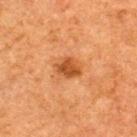Part of a total-body skin-imaging series; this lesion was reviewed on a skin check and was not flagged for biopsy. A close-up tile cropped from a whole-body skin photograph, about 15 mm across. Approximately 3 mm at its widest. Located on the back. The subject is a male in their 50s. Automated tile analysis of the lesion measured an average lesion color of about L≈41 a*≈24 b*≈36 (CIELAB) and a lesion–skin lightness drop of about 10. And it measured a border-irregularity index near 2/10, internal color variation of about 3 on a 0–10 scale, and radial color variation of about 1. And it measured a nevus-likeness score of about 85/100.